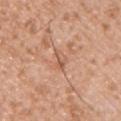Clinical impression:
The lesion was photographed on a routine skin check and not biopsied; there is no pathology result.
Context:
The lesion is located on the chest. A 15 mm crop from a total-body photograph taken for skin-cancer surveillance. A male patient approximately 30 years of age.